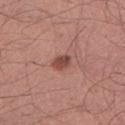<case>
<lesion_size>
  <long_diameter_mm_approx>2.5</long_diameter_mm_approx>
</lesion_size>
<site>left lower leg</site>
<image>
  <source>total-body photography crop</source>
  <field_of_view_mm>15</field_of_view_mm>
</image>
<automated_metrics>
  <eccentricity>0.75</eccentricity>
  <shape_asymmetry>0.15</shape_asymmetry>
  <nevus_likeness_0_100>85</nevus_likeness_0_100>
  <lesion_detection_confidence_0_100>100</lesion_detection_confidence_0_100>
</automated_metrics>
<patient>
  <sex>male</sex>
  <age_approx>40</age_approx>
</patient>
<lighting>white-light</lighting>
</case>